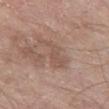Notes:
– workup · no biopsy performed (imaged during a skin exam)
– lighting · white-light illumination
– body site · the left thigh
– automated metrics · roughly 7 lightness units darker than nearby skin and a normalized border contrast of about 5; a detector confidence of about 100 out of 100 that the crop contains a lesion
– patient · male, aged 63–67
– imaging modality · ~15 mm tile from a whole-body skin photo
– size · ≈5 mm